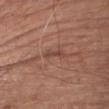Q: Was a biopsy performed?
A: total-body-photography surveillance lesion; no biopsy
Q: How large is the lesion?
A: about 3 mm
Q: What are the patient's age and sex?
A: male, aged approximately 75
Q: What kind of image is this?
A: total-body-photography crop, ~15 mm field of view
Q: Where on the body is the lesion?
A: the chest
Q: Automated lesion metrics?
A: a footprint of about 3 mm², an eccentricity of roughly 0.95, and a shape-asymmetry score of about 0.3 (0 = symmetric); border irregularity of about 3.5 on a 0–10 scale, a within-lesion color-variation index near 0/10, and a peripheral color-asymmetry measure near 0
Q: Illumination type?
A: white-light